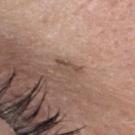Notes:
• follow-up — catalogued during a skin exam; not biopsied
• patient — female, approximately 40 years of age
• anatomic site — the head or neck
• acquisition — ~15 mm crop, total-body skin-cancer survey
• lesion diameter — ~3 mm (longest diameter)
• automated metrics — a lesion area of about 3.5 mm², an outline eccentricity of about 0.9 (0 = round, 1 = elongated), and a shape-asymmetry score of about 0.3 (0 = symmetric); an average lesion color of about L≈49 a*≈17 b*≈24 (CIELAB), a lesion–skin lightness drop of about 8, and a normalized border contrast of about 6; a border-irregularity index near 4/10 and radial color variation of about 0